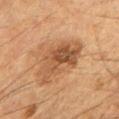Part of a total-body skin-imaging series; this lesion was reviewed on a skin check and was not flagged for biopsy. A close-up tile cropped from a whole-body skin photograph, about 15 mm across. From the right forearm. Automated tile analysis of the lesion measured a shape eccentricity near 0.75. The software also gave about 10 CIELAB-L* units darker than the surrounding skin and a normalized lesion–skin contrast near 7.5. The analysis additionally found border irregularity of about 3.5 on a 0–10 scale, a color-variation rating of about 6.5/10, and a peripheral color-asymmetry measure near 2. And it measured an automated nevus-likeness rating near 15 out of 100 and a detector confidence of about 100 out of 100 that the crop contains a lesion. Captured under cross-polarized illumination. About 6 mm across. The subject is a male aged 83 or older.